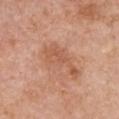{
  "biopsy_status": "not biopsied; imaged during a skin examination",
  "site": "chest",
  "lighting": "white-light",
  "image": {
    "source": "total-body photography crop",
    "field_of_view_mm": 15
  },
  "patient": {
    "sex": "female",
    "age_approx": 60
  }
}